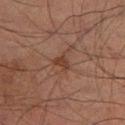Q: Was this lesion biopsied?
A: total-body-photography surveillance lesion; no biopsy
Q: Who is the patient?
A: male, aged 68 to 72
Q: Lesion location?
A: the left lower leg
Q: What did automated image analysis measure?
A: a lesion area of about 3 mm² and an outline eccentricity of about 0.75 (0 = round, 1 = elongated); a classifier nevus-likeness of about 5/100 and a detector confidence of about 100 out of 100 that the crop contains a lesion
Q: What kind of image is this?
A: ~15 mm tile from a whole-body skin photo
Q: What is the lesion's diameter?
A: ~2.5 mm (longest diameter)